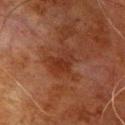The lesion is on the chest.
A roughly 15 mm field-of-view crop from a total-body skin photograph.
The recorded lesion diameter is about 3 mm.
A male patient, about 80 years old.